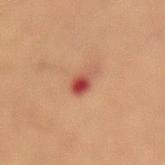Assessment: Part of a total-body skin-imaging series; this lesion was reviewed on a skin check and was not flagged for biopsy. Acquisition and patient details: A 15 mm close-up extracted from a 3D total-body photography capture. This is a cross-polarized tile. On the abdomen. A male subject in their mid-50s. Automated tile analysis of the lesion measured a footprint of about 4.5 mm², a shape eccentricity near 0.7, and a shape-asymmetry score of about 0.3 (0 = symmetric). The analysis additionally found an automated nevus-likeness rating near 0 out of 100 and lesion-presence confidence of about 100/100.